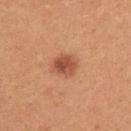  biopsy_status: not biopsied; imaged during a skin examination
  automated_metrics:
    nevus_likeness_0_100: 95
    lesion_detection_confidence_0_100: 100
  lesion_size:
    long_diameter_mm_approx: 3.0
  image:
    source: total-body photography crop
    field_of_view_mm: 15
  site: right upper arm
  lighting: white-light
  patient:
    sex: female
    age_approx: 25